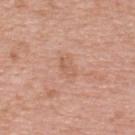The lesion was tiled from a total-body skin photograph and was not biopsied. Measured at roughly 2.5 mm in maximum diameter. Automated image analysis of the tile measured a mean CIELAB color near L≈60 a*≈22 b*≈31 and about 6 CIELAB-L* units darker than the surrounding skin. The software also gave a border-irregularity rating of about 2/10 and internal color variation of about 2 on a 0–10 scale. It also reported a nevus-likeness score of about 0/100 and a lesion-detection confidence of about 100/100. The lesion is on the back. Imaged with white-light lighting. A 15 mm crop from a total-body photograph taken for skin-cancer surveillance. A female subject, aged around 40.